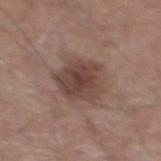From the mid back.
A male patient, about 55 years old.
A close-up tile cropped from a whole-body skin photograph, about 15 mm across.